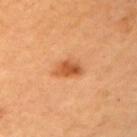Impression:
Captured during whole-body skin photography for melanoma surveillance; the lesion was not biopsied.
Acquisition and patient details:
Approximately 3 mm at its widest. From the left upper arm. A close-up tile cropped from a whole-body skin photograph, about 15 mm across. A female subject, approximately 65 years of age. Captured under cross-polarized illumination.